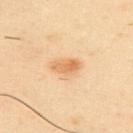The lesion was tiled from a total-body skin photograph and was not biopsied.